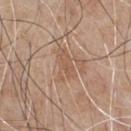The lesion was tiled from a total-body skin photograph and was not biopsied.
The lesion is on the chest.
The patient is a male aged 63–67.
Imaged with white-light lighting.
The lesion's longest dimension is about 4 mm.
A lesion tile, about 15 mm wide, cut from a 3D total-body photograph.
Automated image analysis of the tile measured a footprint of about 6.5 mm², a shape eccentricity near 0.85, and two-axis asymmetry of about 0.35.